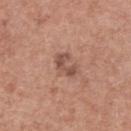biopsy status: imaged on a skin check; not biopsied
acquisition: total-body-photography crop, ~15 mm field of view
subject: female, approximately 60 years of age
location: the upper back